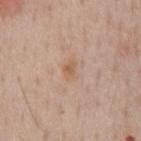No biopsy was performed on this lesion — it was imaged during a full skin examination and was not determined to be concerning. This is a white-light tile. On the chest. A male subject aged 58–62. About 2.5 mm across. A 15 mm close-up tile from a total-body photography series done for melanoma screening.